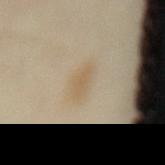workup — total-body-photography surveillance lesion; no biopsy
location — the mid back
lesion size — ~4 mm (longest diameter)
image source — total-body-photography crop, ~15 mm field of view
patient — female, in their 40s